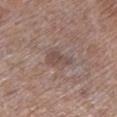biopsy status: total-body-photography surveillance lesion; no biopsy
tile lighting: white-light illumination
location: the leg
lesion diameter: ≈3 mm
patient: female, aged 53 to 57
image source: total-body-photography crop, ~15 mm field of view